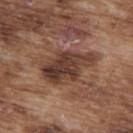Background: A 15 mm close-up extracted from a 3D total-body photography capture. The lesion's longest dimension is about 6.5 mm. Located on the upper back. The tile uses white-light illumination. Automated image analysis of the tile measured a mean CIELAB color near L≈39 a*≈19 b*≈25, roughly 12 lightness units darker than nearby skin, and a normalized lesion–skin contrast near 9.5. The subject is a male approximately 75 years of age.